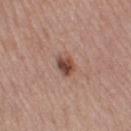This lesion was catalogued during total-body skin photography and was not selected for biopsy.
The subject is a female aged approximately 50.
A 15 mm close-up tile from a total-body photography series done for melanoma screening.
The tile uses white-light illumination.
On the right thigh.